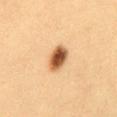{
  "biopsy_status": "not biopsied; imaged during a skin examination",
  "image": {
    "source": "total-body photography crop",
    "field_of_view_mm": 15
  },
  "lesion_size": {
    "long_diameter_mm_approx": 3.5
  },
  "lighting": "cross-polarized",
  "site": "mid back",
  "patient": {
    "sex": "female",
    "age_approx": 30
  }
}